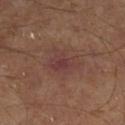Captured during whole-body skin photography for melanoma surveillance; the lesion was not biopsied.
A 15 mm crop from a total-body photograph taken for skin-cancer surveillance.
An algorithmic analysis of the crop reported a nevus-likeness score of about 0/100 and a lesion-detection confidence of about 100/100.
Approximately 5 mm at its widest.
The patient is a male approximately 65 years of age.